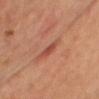  biopsy_status: not biopsied; imaged during a skin examination
  image:
    source: total-body photography crop
    field_of_view_mm: 15
  patient:
    sex: male
    age_approx: 60
  automated_metrics:
    cielab_L: 45
    cielab_a: 27
    cielab_b: 28
    vs_skin_darker_L: 8.0
    vs_skin_contrast_norm: 6.5
    lesion_detection_confidence_0_100: 95
  lighting: cross-polarized
  site: chest
  lesion_size:
    long_diameter_mm_approx: 2.5